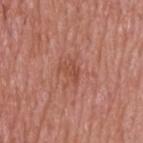Case summary:
* workup: catalogued during a skin exam; not biopsied
* image source: ~15 mm tile from a whole-body skin photo
* image-analysis metrics: a border-irregularity rating of about 6/10 and radial color variation of about 0.5; a lesion-detection confidence of about 100/100
* patient: male, aged around 50
* anatomic site: the upper back
* tile lighting: white-light illumination